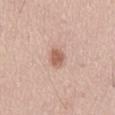* follow-up — no biopsy performed (imaged during a skin exam)
* subject — male, roughly 45 years of age
* body site — the back
* image source — ~15 mm crop, total-body skin-cancer survey
* lighting — white-light